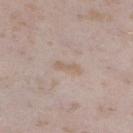Q: Is there a histopathology result?
A: total-body-photography surveillance lesion; no biopsy
Q: Lesion location?
A: the left lower leg
Q: What is the imaging modality?
A: ~15 mm crop, total-body skin-cancer survey
Q: What are the patient's age and sex?
A: female, in their mid- to late 20s
Q: How was the tile lit?
A: white-light illumination